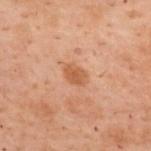biopsy_status: not biopsied; imaged during a skin examination
lesion_size:
  long_diameter_mm_approx: 3.5
patient:
  sex: female
  age_approx: 55
image:
  source: total-body photography crop
  field_of_view_mm: 15
automated_metrics:
  area_mm2_approx: 6.0
  eccentricity: 0.75
  shape_asymmetry: 0.2
site: upper back
lighting: cross-polarized